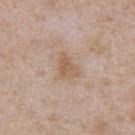Q: Was a biopsy performed?
A: total-body-photography surveillance lesion; no biopsy
Q: What is the anatomic site?
A: the abdomen
Q: What kind of image is this?
A: ~15 mm tile from a whole-body skin photo
Q: Illumination type?
A: white-light illumination
Q: Who is the patient?
A: male, aged around 65